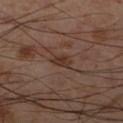<tbp_lesion>
  <biopsy_status>not biopsied; imaged during a skin examination</biopsy_status>
</tbp_lesion>